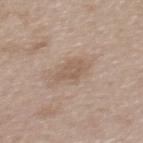Acquisition and patient details: A female patient, aged around 40. Imaged with white-light lighting. On the upper back. A region of skin cropped from a whole-body photographic capture, roughly 15 mm wide.